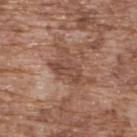Captured during whole-body skin photography for melanoma surveillance; the lesion was not biopsied. A male subject aged approximately 75. Cropped from a whole-body photographic skin survey; the tile spans about 15 mm. The lesion is on the upper back. The lesion's longest dimension is about 6 mm. This is a white-light tile.